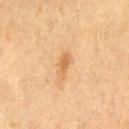Case summary:
- notes — imaged on a skin check; not biopsied
- anatomic site — the left thigh
- patient — female, roughly 40 years of age
- automated metrics — a border-irregularity rating of about 3.5/10, a within-lesion color-variation index near 0.5/10, and radial color variation of about 0; a nevus-likeness score of about 25/100 and a detector confidence of about 100 out of 100 that the crop contains a lesion
- illumination — cross-polarized illumination
- image source — ~15 mm tile from a whole-body skin photo